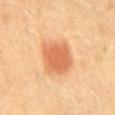notes: catalogued during a skin exam; not biopsied
patient: female, aged 58–62
illumination: cross-polarized
site: the mid back
diameter: about 4.5 mm
acquisition: ~15 mm tile from a whole-body skin photo